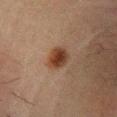The lesion was tiled from a total-body skin photograph and was not biopsied.
About 3 mm across.
The lesion is located on the leg.
A region of skin cropped from a whole-body photographic capture, roughly 15 mm wide.
A male patient, about 50 years old.
The tile uses cross-polarized illumination.